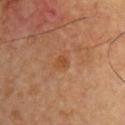Findings:
- size: ≈1.5 mm
- acquisition: 15 mm crop, total-body photography
- patient: male, roughly 65 years of age
- body site: the chest
- lighting: cross-polarized illumination
- pathology: a superficial basal cell carcinoma — a malignant lesion